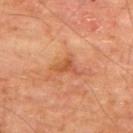No biopsy was performed on this lesion — it was imaged during a full skin examination and was not determined to be concerning. The lesion is on the upper back. Approximately 3 mm at its widest. Imaged with cross-polarized lighting. A male patient, in their mid- to late 60s. A region of skin cropped from a whole-body photographic capture, roughly 15 mm wide. The total-body-photography lesion software estimated a footprint of about 3.5 mm², a shape eccentricity near 0.75, and a symmetry-axis asymmetry near 0.45. It also reported a border-irregularity rating of about 4.5/10, a within-lesion color-variation index near 2/10, and a peripheral color-asymmetry measure near 0.5.